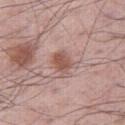follow-up = catalogued during a skin exam; not biopsied | imaging modality = total-body-photography crop, ~15 mm field of view | subject = male, aged around 60 | body site = the leg | lesion size = about 3 mm.